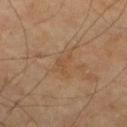notes: no biopsy performed (imaged during a skin exam)
diameter: about 2.5 mm
acquisition: ~15 mm tile from a whole-body skin photo
body site: the left lower leg
patient: male, approximately 70 years of age